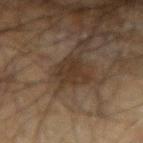No biopsy was performed on this lesion — it was imaged during a full skin examination and was not determined to be concerning.
A close-up tile cropped from a whole-body skin photograph, about 15 mm across.
The subject is a male aged around 70.
On the right forearm.
This is a cross-polarized tile.
About 3.5 mm across.